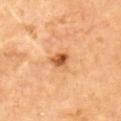Image and clinical context: Captured under cross-polarized illumination. The recorded lesion diameter is about 2.5 mm. On the upper back. A male patient, roughly 70 years of age. A lesion tile, about 15 mm wide, cut from a 3D total-body photograph.